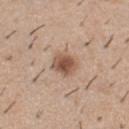Q: Automated lesion metrics?
A: an eccentricity of roughly 0.45 and a shape-asymmetry score of about 0.25 (0 = symmetric); a border-irregularity index near 2/10, internal color variation of about 4 on a 0–10 scale, and radial color variation of about 1
Q: What is the lesion's diameter?
A: ~3 mm (longest diameter)
Q: What are the patient's age and sex?
A: male, in their 40s
Q: What kind of image is this?
A: total-body-photography crop, ~15 mm field of view
Q: Where on the body is the lesion?
A: the front of the torso
Q: How was the tile lit?
A: white-light illumination